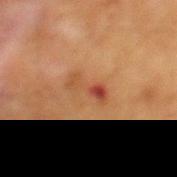No biopsy was performed on this lesion — it was imaged during a full skin examination and was not determined to be concerning.
The recorded lesion diameter is about 4 mm.
Captured under cross-polarized illumination.
On the mid back.
Cropped from a total-body skin-imaging series; the visible field is about 15 mm.
The subject is a male aged approximately 65.